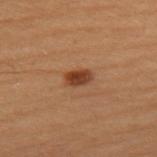| feature | finding |
|---|---|
| follow-up | no biopsy performed (imaged during a skin exam) |
| patient | female, aged approximately 50 |
| location | the left upper arm |
| size | ~3 mm (longest diameter) |
| acquisition | total-body-photography crop, ~15 mm field of view |